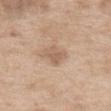workup = catalogued during a skin exam; not biopsied | illumination = white-light illumination | subject = female, aged 73 to 77 | image = ~15 mm crop, total-body skin-cancer survey | TBP lesion metrics = a footprint of about 4.5 mm² and a symmetry-axis asymmetry near 0.25; a border-irregularity rating of about 3/10 and radial color variation of about 1 | diameter = about 3 mm | anatomic site = the mid back.